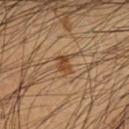Clinical impression:
No biopsy was performed on this lesion — it was imaged during a full skin examination and was not determined to be concerning.
Background:
Located on the chest. Measured at roughly 2 mm in maximum diameter. Imaged with cross-polarized lighting. The subject is a male in their mid-50s. Cropped from a whole-body photographic skin survey; the tile spans about 15 mm.